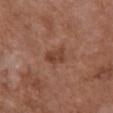{"biopsy_status": "not biopsied; imaged during a skin examination", "lighting": "white-light", "patient": {"sex": "female", "age_approx": 80}, "lesion_size": {"long_diameter_mm_approx": 3.0}, "image": {"source": "total-body photography crop", "field_of_view_mm": 15}, "site": "front of the torso"}